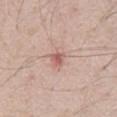The lesion was photographed on a routine skin check and not biopsied; there is no pathology result. A roughly 15 mm field-of-view crop from a total-body skin photograph. The patient is a male about 40 years old. On the chest.